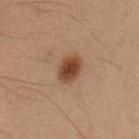| feature | finding |
|---|---|
| workup | no biopsy performed (imaged during a skin exam) |
| image | ~15 mm crop, total-body skin-cancer survey |
| automated metrics | a mean CIELAB color near L≈35 a*≈17 b*≈27 and a lesion-to-skin contrast of about 10 (normalized; higher = more distinct); a nevus-likeness score of about 100/100 and a lesion-detection confidence of about 100/100 |
| patient | male, aged 53 to 57 |
| lighting | cross-polarized |
| lesion diameter | ~3 mm (longest diameter) |
| body site | the left forearm |